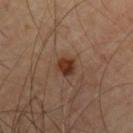Q: Was this lesion biopsied?
A: catalogued during a skin exam; not biopsied
Q: What did automated image analysis measure?
A: an average lesion color of about L≈33 a*≈21 b*≈28 (CIELAB) and roughly 12 lightness units darker than nearby skin; a within-lesion color-variation index near 3.5/10
Q: Patient demographics?
A: male, aged 63 to 67
Q: What lighting was used for the tile?
A: cross-polarized illumination
Q: Where on the body is the lesion?
A: the upper back
Q: How was this image acquired?
A: 15 mm crop, total-body photography